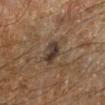Impression: Captured during whole-body skin photography for melanoma surveillance; the lesion was not biopsied. Image and clinical context: The tile uses cross-polarized illumination. Cropped from a whole-body photographic skin survey; the tile spans about 15 mm. The lesion is located on the left lower leg. The lesion's longest dimension is about 3 mm. The subject is a male aged 63–67.